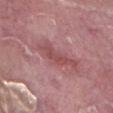* workup: no biopsy performed (imaged during a skin exam)
* imaging modality: ~15 mm crop, total-body skin-cancer survey
* patient: male, aged 38 to 42
* location: the right lower leg
* lesion diameter: ~5.5 mm (longest diameter)
* illumination: white-light